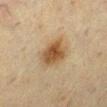The lesion was tiled from a total-body skin photograph and was not biopsied. The lesion-visualizer software estimated a lesion–skin lightness drop of about 10. And it measured border irregularity of about 2.5 on a 0–10 scale, a color-variation rating of about 4.5/10, and peripheral color asymmetry of about 1. And it measured an automated nevus-likeness rating near 95 out of 100 and a detector confidence of about 100 out of 100 that the crop contains a lesion. From the left lower leg. About 4.5 mm across. A female subject, approximately 55 years of age. Captured under cross-polarized illumination. A 15 mm crop from a total-body photograph taken for skin-cancer surveillance.